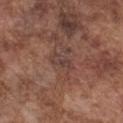Assessment:
Imaged during a routine full-body skin examination; the lesion was not biopsied and no histopathology is available.
Image and clinical context:
Approximately 3 mm at its widest. Automated image analysis of the tile measured a footprint of about 4 mm² and an eccentricity of roughly 0.9. And it measured an average lesion color of about L≈40 a*≈18 b*≈22 (CIELAB), roughly 6 lightness units darker than nearby skin, and a lesion-to-skin contrast of about 6 (normalized; higher = more distinct). The software also gave a border-irregularity index near 3.5/10, a within-lesion color-variation index near 2/10, and peripheral color asymmetry of about 0.5. The software also gave a classifier nevus-likeness of about 0/100 and a lesion-detection confidence of about 95/100. From the chest. The subject is a male roughly 75 years of age. Captured under white-light illumination. A roughly 15 mm field-of-view crop from a total-body skin photograph.